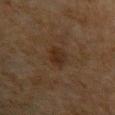biopsy status: catalogued during a skin exam; not biopsied
lesion diameter: about 3 mm
image source: ~15 mm crop, total-body skin-cancer survey
anatomic site: the left upper arm
tile lighting: cross-polarized illumination
subject: male, aged 48–52